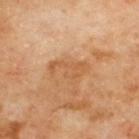workup: catalogued during a skin exam; not biopsied | diameter: ~4.5 mm (longest diameter) | acquisition: 15 mm crop, total-body photography | tile lighting: cross-polarized | patient: male, aged 68 to 72 | site: the upper back.